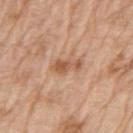workup: imaged on a skin check; not biopsied | body site: the left upper arm | tile lighting: white-light | image source: ~15 mm crop, total-body skin-cancer survey | image-analysis metrics: an area of roughly 5 mm², an outline eccentricity of about 0.9 (0 = round, 1 = elongated), and a shape-asymmetry score of about 0.4 (0 = symmetric); a lesion color around L≈57 a*≈22 b*≈33 in CIELAB, about 10 CIELAB-L* units darker than the surrounding skin, and a normalized border contrast of about 7; a classifier nevus-likeness of about 10/100 and a lesion-detection confidence of about 100/100 | diameter: ~3.5 mm (longest diameter) | subject: male, roughly 70 years of age.